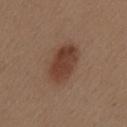follow-up = catalogued during a skin exam; not biopsied | image source = 15 mm crop, total-body photography | patient = female, roughly 40 years of age | lighting = white-light | lesion size = ≈5 mm | site = the mid back.